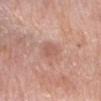The lesion was tiled from a total-body skin photograph and was not biopsied.
About 3 mm across.
Located on the right forearm.
A female patient, aged approximately 65.
The tile uses white-light illumination.
Automated image analysis of the tile measured a footprint of about 4.5 mm² and a shape eccentricity near 0.8.
Cropped from a total-body skin-imaging series; the visible field is about 15 mm.